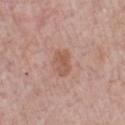follow-up: catalogued during a skin exam; not biopsied
image: 15 mm crop, total-body photography
illumination: white-light illumination
size: ~3 mm (longest diameter)
patient: male, aged 53–57